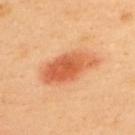This lesion was catalogued during total-body skin photography and was not selected for biopsy. A lesion tile, about 15 mm wide, cut from a 3D total-body photograph. A male subject, roughly 40 years of age. An algorithmic analysis of the crop reported border irregularity of about 2.5 on a 0–10 scale and radial color variation of about 2. And it measured an automated nevus-likeness rating near 100 out of 100 and lesion-presence confidence of about 100/100. Located on the upper back. Captured under cross-polarized illumination. About 6.5 mm across.